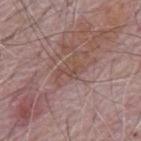notes = no biopsy performed (imaged during a skin exam) | size = about 3.5 mm | tile lighting = white-light illumination | subject = male, aged approximately 65 | body site = the mid back | acquisition = total-body-photography crop, ~15 mm field of view.